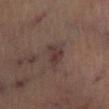workup — total-body-photography surveillance lesion; no biopsy | diameter — ≈3 mm | patient — female, in their 60s | lighting — cross-polarized | acquisition — ~15 mm tile from a whole-body skin photo | body site — the left leg.